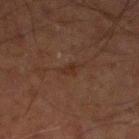Findings:
– workup — imaged on a skin check; not biopsied
– acquisition — total-body-photography crop, ~15 mm field of view
– illumination — cross-polarized
– subject — male, about 70 years old
– anatomic site — the left lower leg